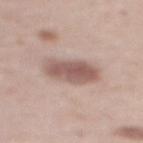<record>
  <site>upper back</site>
  <image>
    <source>total-body photography crop</source>
    <field_of_view_mm>15</field_of_view_mm>
  </image>
  <lighting>white-light</lighting>
  <patient>
    <sex>female</sex>
    <age_approx>65</age_approx>
  </patient>
  <lesion_size>
    <long_diameter_mm_approx>5.0</long_diameter_mm_approx>
  </lesion_size>
</record>